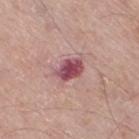{"biopsy_status": "not biopsied; imaged during a skin examination", "automated_metrics": {"area_mm2_approx": 7.5, "eccentricity": 0.8, "shape_asymmetry": 0.2, "cielab_L": 50, "cielab_a": 28, "cielab_b": 17, "vs_skin_darker_L": 15.0, "vs_skin_contrast_norm": 11.0, "border_irregularity_0_10": 2.5, "color_variation_0_10": 6.0, "peripheral_color_asymmetry": 2.0, "nevus_likeness_0_100": 5, "lesion_detection_confidence_0_100": 100}, "lesion_size": {"long_diameter_mm_approx": 4.0}, "image": {"source": "total-body photography crop", "field_of_view_mm": 15}, "lighting": "white-light", "patient": {"sex": "male", "age_approx": 80}, "site": "right thigh"}